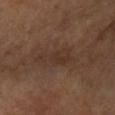<record>
<biopsy_status>not biopsied; imaged during a skin examination</biopsy_status>
<image>
  <source>total-body photography crop</source>
  <field_of_view_mm>15</field_of_view_mm>
</image>
<patient>
  <sex>female</sex>
  <age_approx>65</age_approx>
</patient>
<automated_metrics>
  <eccentricity>0.9</eccentricity>
  <shape_asymmetry>0.45</shape_asymmetry>
  <nevus_likeness_0_100>0</nevus_likeness_0_100>
  <lesion_detection_confidence_0_100>100</lesion_detection_confidence_0_100>
</automated_metrics>
<site>arm</site>
<lesion_size>
  <long_diameter_mm_approx>5.5</long_diameter_mm_approx>
</lesion_size>
</record>